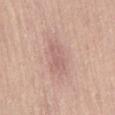workup: no biopsy performed (imaged during a skin exam)
subject: male, roughly 55 years of age
TBP lesion metrics: a lesion area of about 3.5 mm², a shape eccentricity near 0.9, and a shape-asymmetry score of about 0.4 (0 = symmetric); a lesion–skin lightness drop of about 7 and a normalized border contrast of about 4.5; a nevus-likeness score of about 0/100 and a lesion-detection confidence of about 100/100
lesion size: ≈3.5 mm
tile lighting: white-light
image source: ~15 mm tile from a whole-body skin photo